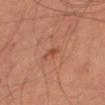Clinical impression: This lesion was catalogued during total-body skin photography and was not selected for biopsy. Clinical summary: The patient is a male roughly 60 years of age. Located on the right thigh. A 15 mm close-up extracted from a 3D total-body photography capture.